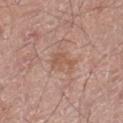Findings:
– workup · catalogued during a skin exam; not biopsied
– subject · male, aged around 55
– image-analysis metrics · a border-irregularity rating of about 6/10, a color-variation rating of about 1/10, and a peripheral color-asymmetry measure near 0.5; a nevus-likeness score of about 0/100 and a detector confidence of about 100 out of 100 that the crop contains a lesion
– location · the left thigh
– illumination · white-light
– acquisition · ~15 mm tile from a whole-body skin photo